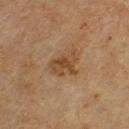Q: Was this lesion biopsied?
A: catalogued during a skin exam; not biopsied
Q: How was the tile lit?
A: cross-polarized
Q: How was this image acquired?
A: ~15 mm tile from a whole-body skin photo
Q: What are the patient's age and sex?
A: male, aged approximately 65
Q: What is the anatomic site?
A: the chest